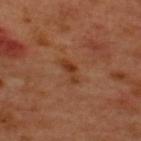{"biopsy_status": "not biopsied; imaged during a skin examination", "image": {"source": "total-body photography crop", "field_of_view_mm": 15}, "lesion_size": {"long_diameter_mm_approx": 3.0}, "site": "upper back", "patient": {"sex": "male", "age_approx": 50}}